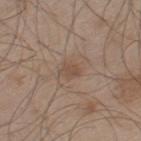<case>
<biopsy_status>not biopsied; imaged during a skin examination</biopsy_status>
<image>
  <source>total-body photography crop</source>
  <field_of_view_mm>15</field_of_view_mm>
</image>
<patient>
  <sex>male</sex>
  <age_approx>60</age_approx>
</patient>
<lighting>white-light</lighting>
<site>left thigh</site>
<lesion_size>
  <long_diameter_mm_approx>2.5</long_diameter_mm_approx>
</lesion_size>
</case>